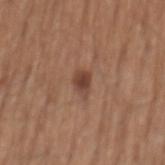• subject — male, in their mid- to late 60s
• image — total-body-photography crop, ~15 mm field of view
• site — the mid back
• size — ~2.5 mm (longest diameter)
• lighting — white-light illumination